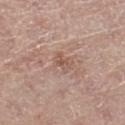The subject is a female in their mid-60s.
A close-up tile cropped from a whole-body skin photograph, about 15 mm across.
Measured at roughly 2 mm in maximum diameter.
On the left thigh.
This is a white-light tile.